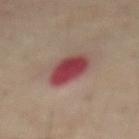follow-up = total-body-photography surveillance lesion; no biopsy
body site = the front of the torso
image = total-body-photography crop, ~15 mm field of view
patient = male, about 70 years old